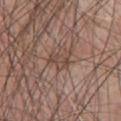{
  "biopsy_status": "not biopsied; imaged during a skin examination",
  "lesion_size": {
    "long_diameter_mm_approx": 3.5
  },
  "site": "front of the torso",
  "automated_metrics": {
    "cielab_L": 46,
    "cielab_a": 17,
    "cielab_b": 24,
    "vs_skin_darker_L": 8.0,
    "vs_skin_contrast_norm": 6.0
  },
  "lighting": "white-light",
  "patient": {
    "sex": "male",
    "age_approx": 60
  },
  "image": {
    "source": "total-body photography crop",
    "field_of_view_mm": 15
  }
}